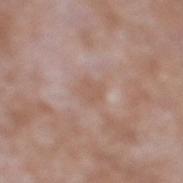Notes:
* notes · no biopsy performed (imaged during a skin exam)
* image-analysis metrics · a mean CIELAB color near L≈57 a*≈18 b*≈26, about 5 CIELAB-L* units darker than the surrounding skin, and a normalized lesion–skin contrast near 4.5
* tile lighting · white-light illumination
* anatomic site · the leg
* subject · male, roughly 60 years of age
* image · total-body-photography crop, ~15 mm field of view